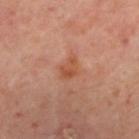• follow-up · catalogued during a skin exam; not biopsied
• patient · aged around 55
• automated metrics · a lesion color around L≈51 a*≈26 b*≈34 in CIELAB, roughly 8 lightness units darker than nearby skin, and a lesion-to-skin contrast of about 7 (normalized; higher = more distinct); a border-irregularity rating of about 2/10 and a within-lesion color-variation index near 3.5/10; a nevus-likeness score of about 5/100 and a detector confidence of about 100 out of 100 that the crop contains a lesion
• acquisition · 15 mm crop, total-body photography
• anatomic site · the upper back
• size · ~2.5 mm (longest diameter)